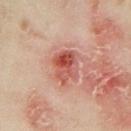Assessment: No biopsy was performed on this lesion — it was imaged during a full skin examination and was not determined to be concerning. Clinical summary: On the arm. A close-up tile cropped from a whole-body skin photograph, about 15 mm across. A female subject aged around 35. Automated image analysis of the tile measured a lesion area of about 9 mm² and a symmetry-axis asymmetry near 0.3. And it measured a border-irregularity index near 3/10 and radial color variation of about 3.5. The software also gave an automated nevus-likeness rating near 0 out of 100. Approximately 4 mm at its widest.